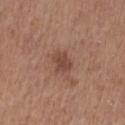{"biopsy_status": "not biopsied; imaged during a skin examination", "patient": {"sex": "male", "age_approx": 65}, "automated_metrics": {"cielab_L": 45, "cielab_a": 21, "cielab_b": 27, "vs_skin_darker_L": 8.0, "vs_skin_contrast_norm": 6.5, "border_irregularity_0_10": 2.0, "nevus_likeness_0_100": 10, "lesion_detection_confidence_0_100": 100}, "site": "chest", "lesion_size": {"long_diameter_mm_approx": 2.5}, "image": {"source": "total-body photography crop", "field_of_view_mm": 15}}